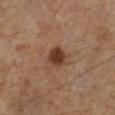Captured during whole-body skin photography for melanoma surveillance; the lesion was not biopsied.
A roughly 15 mm field-of-view crop from a total-body skin photograph.
A male patient approximately 70 years of age.
On the left lower leg.